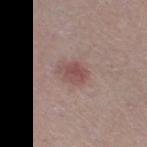follow-up=no biopsy performed (imaged during a skin exam)
patient=female, approximately 40 years of age
image=total-body-photography crop, ~15 mm field of view
site=the right thigh
TBP lesion metrics=an automated nevus-likeness rating near 55 out of 100